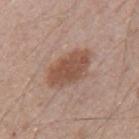The lesion was tiled from a total-body skin photograph and was not biopsied.
This image is a 15 mm lesion crop taken from a total-body photograph.
Located on the upper back.
This is a white-light tile.
The total-body-photography lesion software estimated an eccentricity of roughly 0.85 and a symmetry-axis asymmetry near 0.15. It also reported a lesion color around L≈51 a*≈20 b*≈27 in CIELAB and a normalized lesion–skin contrast near 8. The software also gave a within-lesion color-variation index near 2.5/10 and peripheral color asymmetry of about 1. It also reported a classifier nevus-likeness of about 75/100.
A male patient roughly 40 years of age.
The lesion's longest dimension is about 5.5 mm.